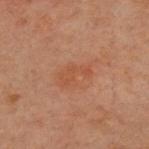imaging modality = ~15 mm tile from a whole-body skin photo; site = the chest; size = about 3.5 mm; subject = male, aged approximately 60; lighting = cross-polarized illumination.